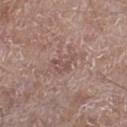<record>
  <biopsy_status>not biopsied; imaged during a skin examination</biopsy_status>
  <automated_metrics>
    <area_mm2_approx>5.0</area_mm2_approx>
    <eccentricity>0.8</eccentricity>
    <shape_asymmetry>0.45</shape_asymmetry>
    <nevus_likeness_0_100>0</nevus_likeness_0_100>
    <lesion_detection_confidence_0_100>95</lesion_detection_confidence_0_100>
  </automated_metrics>
  <image>
    <source>total-body photography crop</source>
    <field_of_view_mm>15</field_of_view_mm>
  </image>
  <lesion_size>
    <long_diameter_mm_approx>3.5</long_diameter_mm_approx>
  </lesion_size>
  <site>right lower leg</site>
  <patient>
    <sex>male</sex>
    <age_approx>80</age_approx>
  </patient>
</record>